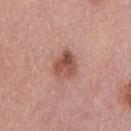| key | value |
|---|---|
| workup | total-body-photography surveillance lesion; no biopsy |
| image | total-body-photography crop, ~15 mm field of view |
| automated lesion analysis | a footprint of about 7.5 mm² and a symmetry-axis asymmetry near 0.25; an average lesion color of about L≈52 a*≈24 b*≈28 (CIELAB), a lesion–skin lightness drop of about 12, and a normalized border contrast of about 8.5; border irregularity of about 2 on a 0–10 scale, internal color variation of about 7 on a 0–10 scale, and a peripheral color-asymmetry measure near 2.5 |
| subject | male, roughly 30 years of age |
| lesion diameter | about 3 mm |